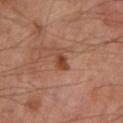Assessment: The lesion was tiled from a total-body skin photograph and was not biopsied. Image and clinical context: A male subject aged 68–72. A region of skin cropped from a whole-body photographic capture, roughly 15 mm wide. The lesion is located on the left lower leg.